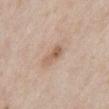This is a white-light tile.
Longest diameter approximately 3 mm.
The subject is a male aged approximately 65.
An algorithmic analysis of the crop reported a footprint of about 4 mm², an outline eccentricity of about 0.85 (0 = round, 1 = elongated), and a symmetry-axis asymmetry near 0.35. The software also gave a mean CIELAB color near L≈59 a*≈18 b*≈29, roughly 10 lightness units darker than nearby skin, and a lesion-to-skin contrast of about 6.5 (normalized; higher = more distinct). It also reported a classifier nevus-likeness of about 20/100 and a lesion-detection confidence of about 100/100.
This image is a 15 mm lesion crop taken from a total-body photograph.
On the abdomen.